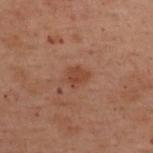<lesion>
  <biopsy_status>not biopsied; imaged during a skin examination</biopsy_status>
  <image>
    <source>total-body photography crop</source>
    <field_of_view_mm>15</field_of_view_mm>
  </image>
  <lesion_size>
    <long_diameter_mm_approx>2.5</long_diameter_mm_approx>
  </lesion_size>
  <lighting>cross-polarized</lighting>
  <patient>
    <sex>female</sex>
    <age_approx>55</age_approx>
  </patient>
  <site>upper back</site>
</lesion>